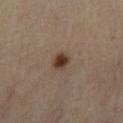notes=no biopsy performed (imaged during a skin exam)
imaging modality=15 mm crop, total-body photography
body site=the right lower leg
patient=male, aged 53–57
TBP lesion metrics=a lesion area of about 4.5 mm² and a symmetry-axis asymmetry near 0.25; a lesion color around L≈38 a*≈16 b*≈26 in CIELAB; internal color variation of about 4.5 on a 0–10 scale and peripheral color asymmetry of about 1.5
lighting=cross-polarized illumination
lesion diameter=≈2.5 mm